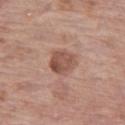The lesion was tiled from a total-body skin photograph and was not biopsied.
Measured at roughly 3.5 mm in maximum diameter.
On the leg.
A female patient, aged approximately 85 or older.
Cropped from a total-body skin-imaging series; the visible field is about 15 mm.